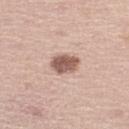biopsy status: catalogued during a skin exam; not biopsied
image source: total-body-photography crop, ~15 mm field of view
illumination: white-light
subject: male, aged 63 to 67
anatomic site: the leg
lesion size: ≈3.5 mm